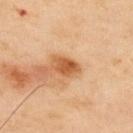The lesion was photographed on a routine skin check and not biopsied; there is no pathology result. The lesion-visualizer software estimated a lesion area of about 4.5 mm² and a shape eccentricity near 0.7. The software also gave radial color variation of about 1. The analysis additionally found a nevus-likeness score of about 95/100 and a lesion-detection confidence of about 100/100. This image is a 15 mm lesion crop taken from a total-body photograph. A male patient, aged 53–57. Located on the upper back. Imaged with cross-polarized lighting. Measured at roughly 3 mm in maximum diameter.